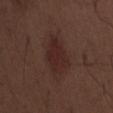Part of a total-body skin-imaging series; this lesion was reviewed on a skin check and was not flagged for biopsy. The lesion is located on the abdomen. Cropped from a total-body skin-imaging series; the visible field is about 15 mm. The patient is a male aged 68–72. Imaged with white-light lighting.